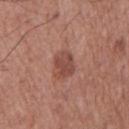Clinical impression: Imaged during a routine full-body skin examination; the lesion was not biopsied and no histopathology is available. Clinical summary: Longest diameter approximately 3 mm. The tile uses white-light illumination. The total-body-photography lesion software estimated a classifier nevus-likeness of about 10/100. On the chest. A male subject in their mid-70s. A 15 mm close-up tile from a total-body photography series done for melanoma screening.